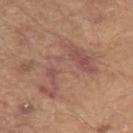Assessment: Imaged during a routine full-body skin examination; the lesion was not biopsied and no histopathology is available. Acquisition and patient details: A male subject, approximately 80 years of age. Located on the left upper arm. Longest diameter approximately 8 mm. An algorithmic analysis of the crop reported a footprint of about 18 mm², an outline eccentricity of about 0.85 (0 = round, 1 = elongated), and a shape-asymmetry score of about 0.7 (0 = symmetric). And it measured peripheral color asymmetry of about 1. And it measured an automated nevus-likeness rating near 0 out of 100 and a lesion-detection confidence of about 95/100. A roughly 15 mm field-of-view crop from a total-body skin photograph.